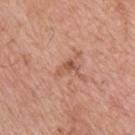Notes:
– biopsy status — imaged on a skin check; not biopsied
– image source — 15 mm crop, total-body photography
– location — the back
– subject — male, about 75 years old